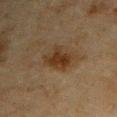Image and clinical context:
Imaged with cross-polarized lighting. A region of skin cropped from a whole-body photographic capture, roughly 15 mm wide. Approximately 4 mm at its widest. From the left upper arm. A male subject aged around 65.